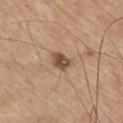This lesion was catalogued during total-body skin photography and was not selected for biopsy.
Longest diameter approximately 3 mm.
A 15 mm close-up extracted from a 3D total-body photography capture.
The patient is a male in their mid- to late 70s.
From the mid back.